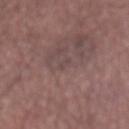This lesion was catalogued during total-body skin photography and was not selected for biopsy. The subject is a male aged 63–67. Cropped from a total-body skin-imaging series; the visible field is about 15 mm. From the abdomen.